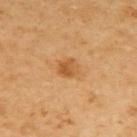notes — imaged on a skin check; not biopsied | subject — female, aged approximately 55 | site — the back | lesion diameter — about 2.5 mm | image-analysis metrics — an area of roughly 4.5 mm², a shape eccentricity near 0.45, and a symmetry-axis asymmetry near 0.15 | imaging modality — 15 mm crop, total-body photography.